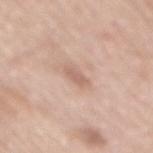Notes:
• follow-up · no biopsy performed (imaged during a skin exam)
• subject · male, aged approximately 70
• image source · 15 mm crop, total-body photography
• site · the mid back